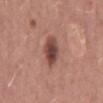No biopsy was performed on this lesion — it was imaged during a full skin examination and was not determined to be concerning.
The recorded lesion diameter is about 4.5 mm.
The lesion is on the mid back.
Cropped from a whole-body photographic skin survey; the tile spans about 15 mm.
A male subject, in their 50s.
Imaged with white-light lighting.